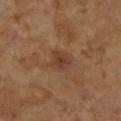– follow-up: catalogued during a skin exam; not biopsied
– size: ~3 mm (longest diameter)
– lighting: cross-polarized
– acquisition: 15 mm crop, total-body photography
– anatomic site: the left forearm
– subject: female, roughly 70 years of age
– image-analysis metrics: a lesion area of about 5 mm² and two-axis asymmetry of about 0.2; a border-irregularity index near 2/10, a color-variation rating of about 3/10, and a peripheral color-asymmetry measure near 1